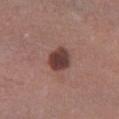Clinical impression: The lesion was tiled from a total-body skin photograph and was not biopsied. Acquisition and patient details: Longest diameter approximately 3 mm. A roughly 15 mm field-of-view crop from a total-body skin photograph. Located on the left lower leg. The lesion-visualizer software estimated border irregularity of about 1.5 on a 0–10 scale, a color-variation rating of about 3.5/10, and peripheral color asymmetry of about 1. This is a white-light tile. The patient is a female aged around 60.